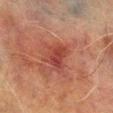Q: Is there a histopathology result?
A: catalogued during a skin exam; not biopsied
Q: What lighting was used for the tile?
A: cross-polarized illumination
Q: Where on the body is the lesion?
A: the left lower leg
Q: How was this image acquired?
A: ~15 mm tile from a whole-body skin photo
Q: What are the patient's age and sex?
A: male, about 70 years old
Q: What did automated image analysis measure?
A: an eccentricity of roughly 0.65 and two-axis asymmetry of about 0.35; border irregularity of about 3.5 on a 0–10 scale, a color-variation rating of about 4.5/10, and radial color variation of about 1.5